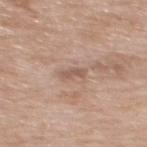notes: no biopsy performed (imaged during a skin exam); acquisition: 15 mm crop, total-body photography; site: the upper back; subject: female, aged around 65.